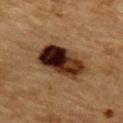<case>
  <biopsy_status>not biopsied; imaged during a skin examination</biopsy_status>
  <patient>
    <sex>male</sex>
    <age_approx>85+</age_approx>
  </patient>
  <lighting>cross-polarized</lighting>
  <image>
    <source>total-body photography crop</source>
    <field_of_view_mm>15</field_of_view_mm>
  </image>
  <lesion_size>
    <long_diameter_mm_approx>7.0</long_diameter_mm_approx>
  </lesion_size>
  <site>mid back</site>
  <automated_metrics>
    <cielab_L>25</cielab_L>
    <cielab_a>17</cielab_a>
    <cielab_b>24</cielab_b>
    <vs_skin_darker_L>18.0</vs_skin_darker_L>
    <vs_skin_contrast_norm>17.5</vs_skin_contrast_norm>
  </automated_metrics>
</case>